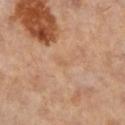{
  "biopsy_status": "not biopsied; imaged during a skin examination",
  "lighting": "cross-polarized",
  "image": {
    "source": "total-body photography crop",
    "field_of_view_mm": 15
  },
  "lesion_size": {
    "long_diameter_mm_approx": 1.0
  },
  "site": "right lower leg",
  "patient": {
    "sex": "female",
    "age_approx": 60
  }
}